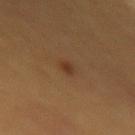Imaged during a routine full-body skin examination; the lesion was not biopsied and no histopathology is available.
The lesion is on the right thigh.
Imaged with cross-polarized lighting.
The subject is a male aged 58 to 62.
A 15 mm close-up tile from a total-body photography series done for melanoma screening.
About 2 mm across.